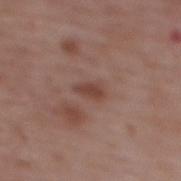biopsy status: total-body-photography surveillance lesion; no biopsy
imaging modality: ~15 mm tile from a whole-body skin photo
subject: female, aged approximately 65
location: the upper back